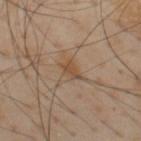notes: total-body-photography surveillance lesion; no biopsy
location: the upper back
subject: male, aged 53–57
lesion diameter: about 3 mm
imaging modality: ~15 mm crop, total-body skin-cancer survey
lighting: cross-polarized illumination
image-analysis metrics: a lesion area of about 3.5 mm² and an outline eccentricity of about 0.8 (0 = round, 1 = elongated); a mean CIELAB color near L≈49 a*≈16 b*≈31, a lesion–skin lightness drop of about 8, and a lesion-to-skin contrast of about 7 (normalized; higher = more distinct); border irregularity of about 3 on a 0–10 scale, internal color variation of about 2 on a 0–10 scale, and radial color variation of about 1